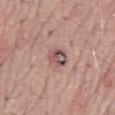Assessment: The lesion was tiled from a total-body skin photograph and was not biopsied. Context: A region of skin cropped from a whole-body photographic capture, roughly 15 mm wide. The lesion is on the mid back. A male patient, approximately 70 years of age.